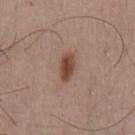Findings:
* biopsy status · total-body-photography surveillance lesion; no biopsy
* patient · male, aged approximately 55
* tile lighting · white-light illumination
* anatomic site · the chest
* acquisition · total-body-photography crop, ~15 mm field of view